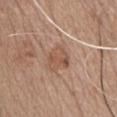• notes: imaged on a skin check; not biopsied
• site: the front of the torso
• illumination: white-light illumination
• acquisition: ~15 mm tile from a whole-body skin photo
• lesion diameter: ≈3.5 mm
• patient: male, aged 63–67
• TBP lesion metrics: a lesion color around L≈53 a*≈20 b*≈30 in CIELAB, roughly 8 lightness units darker than nearby skin, and a normalized border contrast of about 6; border irregularity of about 3 on a 0–10 scale, a color-variation rating of about 3/10, and peripheral color asymmetry of about 1